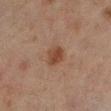– notes — catalogued during a skin exam; not biopsied
– site — the right lower leg
– image-analysis metrics — a footprint of about 5 mm² and a shape eccentricity near 0.65; an automated nevus-likeness rating near 95 out of 100
– imaging modality — total-body-photography crop, ~15 mm field of view
– diameter — about 3 mm
– patient — male, in their mid-60s
– tile lighting — cross-polarized